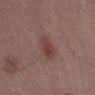Recorded during total-body skin imaging; not selected for excision or biopsy.